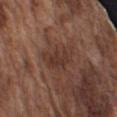The lesion was photographed on a routine skin check and not biopsied; there is no pathology result.
This is a white-light tile.
On the arm.
A male patient, roughly 75 years of age.
Measured at roughly 5 mm in maximum diameter.
Automated tile analysis of the lesion measured an area of roughly 9.5 mm², an eccentricity of roughly 0.85, and a shape-asymmetry score of about 0.35 (0 = symmetric). The analysis additionally found a border-irregularity index near 4.5/10, internal color variation of about 2.5 on a 0–10 scale, and radial color variation of about 1. The software also gave a classifier nevus-likeness of about 5/100.
A lesion tile, about 15 mm wide, cut from a 3D total-body photograph.